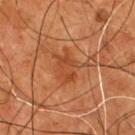Clinical impression: The lesion was tiled from a total-body skin photograph and was not biopsied. Background: Captured under cross-polarized illumination. The lesion is located on the chest. Automated tile analysis of the lesion measured a footprint of about 9 mm², an eccentricity of roughly 0.5, and a symmetry-axis asymmetry near 0.35. A male subject in their mid- to late 50s. The lesion's longest dimension is about 4 mm. A roughly 15 mm field-of-view crop from a total-body skin photograph.